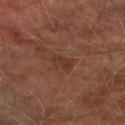Q: Is there a histopathology result?
A: total-body-photography surveillance lesion; no biopsy
Q: Where on the body is the lesion?
A: the leg
Q: What did automated image analysis measure?
A: a classifier nevus-likeness of about 5/100 and a detector confidence of about 100 out of 100 that the crop contains a lesion
Q: What is the imaging modality?
A: ~15 mm crop, total-body skin-cancer survey
Q: What lighting was used for the tile?
A: cross-polarized
Q: What are the patient's age and sex?
A: male, roughly 75 years of age